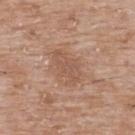workup: no biopsy performed (imaged during a skin exam) | image-analysis metrics: an area of roughly 8 mm² and an eccentricity of roughly 0.85; about 7 CIELAB-L* units darker than the surrounding skin and a lesion-to-skin contrast of about 5 (normalized; higher = more distinct); a border-irregularity index near 3.5/10, internal color variation of about 2 on a 0–10 scale, and peripheral color asymmetry of about 0.5; a classifier nevus-likeness of about 0/100 and a detector confidence of about 100 out of 100 that the crop contains a lesion | subject: male, aged 48–52 | site: the back | illumination: white-light illumination | imaging modality: total-body-photography crop, ~15 mm field of view | size: ~4 mm (longest diameter).